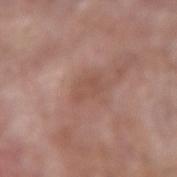Clinical impression:
Recorded during total-body skin imaging; not selected for excision or biopsy.
Context:
This is a white-light tile. The recorded lesion diameter is about 3 mm. A male patient, in their mid- to late 50s. The lesion is on the left forearm. A region of skin cropped from a whole-body photographic capture, roughly 15 mm wide.